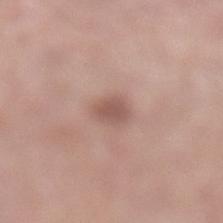Imaged during a routine full-body skin examination; the lesion was not biopsied and no histopathology is available. This is a white-light tile. A male subject about 55 years old. From the left lower leg. A lesion tile, about 15 mm wide, cut from a 3D total-body photograph. Longest diameter approximately 2.5 mm.